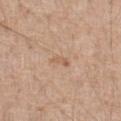The lesion was tiled from a total-body skin photograph and was not biopsied. Imaged with white-light lighting. Cropped from a total-body skin-imaging series; the visible field is about 15 mm. From the front of the torso. A male subject aged around 70.